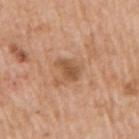biopsy status: total-body-photography surveillance lesion; no biopsy | location: the right upper arm | patient: male, in their 60s | illumination: white-light illumination | imaging modality: ~15 mm crop, total-body skin-cancer survey | automated metrics: a shape eccentricity near 0.8; a lesion color around L≈53 a*≈21 b*≈34 in CIELAB, roughly 10 lightness units darker than nearby skin, and a lesion-to-skin contrast of about 7 (normalized; higher = more distinct); border irregularity of about 2.5 on a 0–10 scale and peripheral color asymmetry of about 1; an automated nevus-likeness rating near 25 out of 100 and a detector confidence of about 100 out of 100 that the crop contains a lesion | size: ≈3 mm.